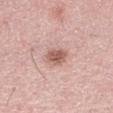The total-body-photography lesion software estimated radial color variation of about 1. And it measured a nevus-likeness score of about 70/100 and a lesion-detection confidence of about 100/100.
The lesion is located on the right thigh.
The patient is a male in their 50s.
A lesion tile, about 15 mm wide, cut from a 3D total-body photograph.